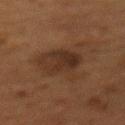follow-up = imaged on a skin check; not biopsied
size = about 6.5 mm
image source = 15 mm crop, total-body photography
tile lighting = cross-polarized illumination
patient = female, aged approximately 50
anatomic site = the mid back